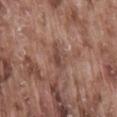Clinical impression:
Recorded during total-body skin imaging; not selected for excision or biopsy.
Background:
Approximately 3 mm at its widest. A male patient, aged 73 to 77. A roughly 15 mm field-of-view crop from a total-body skin photograph. From the lower back. Imaged with white-light lighting. Automated tile analysis of the lesion measured an average lesion color of about L≈45 a*≈20 b*≈24 (CIELAB) and roughly 8 lightness units darker than nearby skin.